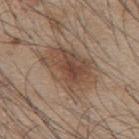Q: Is there a histopathology result?
A: no biopsy performed (imaged during a skin exam)
Q: Lesion location?
A: the upper back
Q: What kind of image is this?
A: 15 mm crop, total-body photography
Q: What is the lesion's diameter?
A: ≈7 mm
Q: What lighting was used for the tile?
A: white-light
Q: Patient demographics?
A: male, roughly 45 years of age